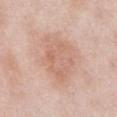Impression: Imaged during a routine full-body skin examination; the lesion was not biopsied and no histopathology is available. Acquisition and patient details: The lesion-visualizer software estimated a lesion area of about 18 mm², a shape eccentricity near 0.65, and a symmetry-axis asymmetry near 0.25. And it measured a lesion color around L≈65 a*≈21 b*≈29 in CIELAB, roughly 7 lightness units darker than nearby skin, and a normalized border contrast of about 5. The analysis additionally found a border-irregularity index near 3.5/10, a within-lesion color-variation index near 3/10, and peripheral color asymmetry of about 1. The analysis additionally found a classifier nevus-likeness of about 5/100 and a detector confidence of about 100 out of 100 that the crop contains a lesion. A roughly 15 mm field-of-view crop from a total-body skin photograph. Approximately 6 mm at its widest. The subject is a male approximately 55 years of age. This is a white-light tile. On the abdomen.